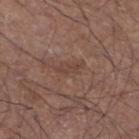Assessment: The lesion was tiled from a total-body skin photograph and was not biopsied. Context: Located on the left lower leg. Imaged with white-light lighting. A 15 mm close-up extracted from a 3D total-body photography capture. The patient is a male aged 58–62. Longest diameter approximately 2.5 mm.